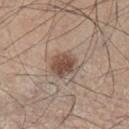Clinical summary: Located on the left lower leg. A roughly 15 mm field-of-view crop from a total-body skin photograph. The tile uses white-light illumination. Longest diameter approximately 3.5 mm. Automated tile analysis of the lesion measured a footprint of about 8.5 mm², a shape eccentricity near 0.5, and two-axis asymmetry of about 0.15. A male patient, about 45 years old.